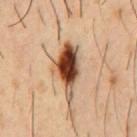notes: imaged on a skin check; not biopsied
location: the chest
lesion size: ≈7 mm
image-analysis metrics: an area of roughly 14 mm², an outline eccentricity of about 0.85 (0 = round, 1 = elongated), and a symmetry-axis asymmetry near 0.35; a border-irregularity rating of about 4/10, internal color variation of about 10 on a 0–10 scale, and peripheral color asymmetry of about 4
image: total-body-photography crop, ~15 mm field of view
patient: male, in their mid-50s
illumination: cross-polarized illumination